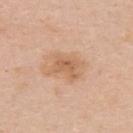notes=imaged on a skin check; not biopsied
location=the upper back
illumination=white-light illumination
imaging modality=15 mm crop, total-body photography
size=about 3.5 mm
image-analysis metrics=a shape eccentricity near 0.7 and a symmetry-axis asymmetry near 0.25; a border-irregularity index near 3/10 and a peripheral color-asymmetry measure near 1; an automated nevus-likeness rating near 5 out of 100 and a detector confidence of about 100 out of 100 that the crop contains a lesion
subject=female, in their mid-30s